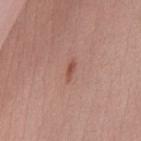Findings:
• notes · catalogued during a skin exam; not biopsied
• tile lighting · white-light
• patient · female, roughly 25 years of age
• acquisition · ~15 mm tile from a whole-body skin photo
• automated metrics · a footprint of about 2 mm², an eccentricity of roughly 0.95, and a shape-asymmetry score of about 0.35 (0 = symmetric)
• lesion size · ≈2.5 mm
• anatomic site · the chest